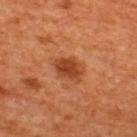The lesion was tiled from a total-body skin photograph and was not biopsied. Cropped from a total-body skin-imaging series; the visible field is about 15 mm. On the upper back. The patient is a male aged approximately 65.